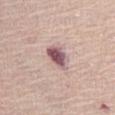Clinical impression:
Captured during whole-body skin photography for melanoma surveillance; the lesion was not biopsied.
Context:
From the left thigh. A female patient aged 68–72. Imaged with white-light lighting. An algorithmic analysis of the crop reported an eccentricity of roughly 0.7 and a shape-asymmetry score of about 0.25 (0 = symmetric). The software also gave a nevus-likeness score of about 5/100. Cropped from a whole-body photographic skin survey; the tile spans about 15 mm.